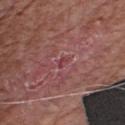<lesion>
  <lesion_size>
    <long_diameter_mm_approx>1.5</long_diameter_mm_approx>
  </lesion_size>
  <image>
    <source>total-body photography crop</source>
    <field_of_view_mm>15</field_of_view_mm>
  </image>
  <automated_metrics>
    <eccentricity>0.8</eccentricity>
    <shape_asymmetry>0.3</shape_asymmetry>
    <cielab_L>41</cielab_L>
    <cielab_a>29</cielab_a>
    <cielab_b>20</cielab_b>
    <vs_skin_darker_L>7.0</vs_skin_darker_L>
    <border_irregularity_0_10>3.0</border_irregularity_0_10>
    <color_variation_0_10>0.0</color_variation_0_10>
  </automated_metrics>
  <patient>
    <sex>male</sex>
    <age_approx>75</age_approx>
  </patient>
  <lighting>white-light</lighting>
  <site>front of the torso</site>
  <diagnosis>
    <histopathology>nodular basal cell carcinoma</histopathology>
    <malignancy>malignant</malignancy>
    <taxonomic_path>Malignant; Malignant adnexal epithelial proliferations - Follicular; Basal cell carcinoma; Basal cell carcinoma, Nodular</taxonomic_path>
  </diagnosis>
</lesion>